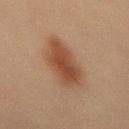<lesion>
<automated_metrics>
  <area_mm2_approx>19.0</area_mm2_approx>
  <eccentricity>0.9</eccentricity>
  <shape_asymmetry>0.2</shape_asymmetry>
  <color_variation_0_10>3.5</color_variation_0_10>
  <peripheral_color_asymmetry>1.0</peripheral_color_asymmetry>
  <nevus_likeness_0_100>100</nevus_likeness_0_100>
</automated_metrics>
<lesion_size>
  <long_diameter_mm_approx>7.5</long_diameter_mm_approx>
</lesion_size>
<site>mid back</site>
<lighting>cross-polarized</lighting>
<image>
  <source>total-body photography crop</source>
  <field_of_view_mm>15</field_of_view_mm>
</image>
<patient>
  <sex>male</sex>
  <age_approx>60</age_approx>
</patient>
</lesion>